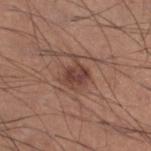follow-up = imaged on a skin check; not biopsied | site = the leg | patient = male, aged 33 to 37 | imaging modality = total-body-photography crop, ~15 mm field of view | tile lighting = white-light illumination | size = about 3.5 mm.